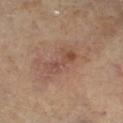Clinical impression:
No biopsy was performed on this lesion — it was imaged during a full skin examination and was not determined to be concerning.
Clinical summary:
A 15 mm crop from a total-body photograph taken for skin-cancer surveillance. A female subject aged 58–62. The lesion is located on the leg. Measured at roughly 4 mm in maximum diameter. Captured under cross-polarized illumination.